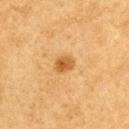<case>
  <biopsy_status>not biopsied; imaged during a skin examination</biopsy_status>
  <lesion_size>
    <long_diameter_mm_approx>2.5</long_diameter_mm_approx>
  </lesion_size>
  <lighting>cross-polarized</lighting>
  <image>
    <source>total-body photography crop</source>
    <field_of_view_mm>15</field_of_view_mm>
  </image>
  <patient>
    <sex>male</sex>
    <age_approx>55</age_approx>
  </patient>
  <site>arm</site>
</case>